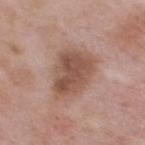The lesion was photographed on a routine skin check and not biopsied; there is no pathology result. A male subject approximately 55 years of age. The lesion is located on the upper back. A 15 mm close-up extracted from a 3D total-body photography capture.